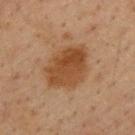notes: no biopsy performed (imaged during a skin exam)
tile lighting: cross-polarized
body site: the upper back
patient: male, in their mid-30s
lesion diameter: about 6 mm
image: ~15 mm tile from a whole-body skin photo